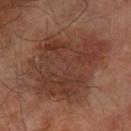Q: Is there a histopathology result?
A: imaged on a skin check; not biopsied
Q: Illumination type?
A: cross-polarized
Q: Lesion location?
A: the right forearm
Q: What are the patient's age and sex?
A: male, in their mid-60s
Q: What is the imaging modality?
A: ~15 mm tile from a whole-body skin photo